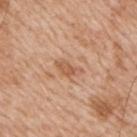automated lesion analysis = a border-irregularity index near 4/10 and internal color variation of about 2 on a 0–10 scale; a nevus-likeness score of about 0/100 and a lesion-detection confidence of about 100/100
patient = male, roughly 65 years of age
illumination = white-light illumination
body site = the right upper arm
imaging modality = 15 mm crop, total-body photography
lesion diameter = ~3.5 mm (longest diameter)